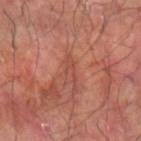  biopsy_status: not biopsied; imaged during a skin examination
  site: left forearm
  image:
    source: total-body photography crop
    field_of_view_mm: 15
  lesion_size:
    long_diameter_mm_approx: 3.0
  patient:
    sex: male
    age_approx: 65